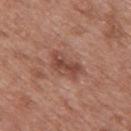No biopsy was performed on this lesion — it was imaged during a full skin examination and was not determined to be concerning. A male subject aged 48 to 52. A roughly 15 mm field-of-view crop from a total-body skin photograph. The lesion is located on the back. The tile uses white-light illumination.